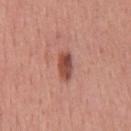<record>
  <biopsy_status>not biopsied; imaged during a skin examination</biopsy_status>
  <site>upper back</site>
  <patient>
    <sex>male</sex>
    <age_approx>45</age_approx>
  </patient>
  <image>
    <source>total-body photography crop</source>
    <field_of_view_mm>15</field_of_view_mm>
  </image>
  <lighting>white-light</lighting>
</record>